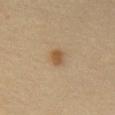Impression:
This lesion was catalogued during total-body skin photography and was not selected for biopsy.
Background:
The patient is a male aged around 50. Imaged with cross-polarized lighting. The lesion is on the chest. Longest diameter approximately 2.5 mm. Automated tile analysis of the lesion measured an average lesion color of about L≈53 a*≈17 b*≈35 (CIELAB), roughly 10 lightness units darker than nearby skin, and a normalized lesion–skin contrast near 7.5. And it measured a border-irregularity rating of about 2/10, a within-lesion color-variation index near 1/10, and a peripheral color-asymmetry measure near 0.5. This image is a 15 mm lesion crop taken from a total-body photograph.